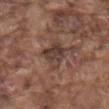| field | value |
|---|---|
| biopsy status | catalogued during a skin exam; not biopsied |
| patient | male, aged 73 to 77 |
| diameter | about 3.5 mm |
| illumination | white-light illumination |
| location | the mid back |
| imaging modality | ~15 mm tile from a whole-body skin photo |